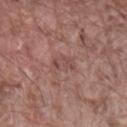biopsy_status: not biopsied; imaged during a skin examination
site: lower back
automated_metrics:
  area_mm2_approx: 3.5
  cielab_L: 47
  cielab_a: 21
  cielab_b: 22
  vs_skin_darker_L: 7.0
  vs_skin_contrast_norm: 5.5
  color_variation_0_10: 0.0
  peripheral_color_asymmetry: 0.0
lesion_size:
  long_diameter_mm_approx: 3.0
image:
  source: total-body photography crop
  field_of_view_mm: 15
lighting: white-light
patient:
  sex: male
  age_approx: 80